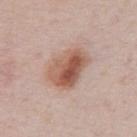{
  "biopsy_status": "not biopsied; imaged during a skin examination",
  "image": {
    "source": "total-body photography crop",
    "field_of_view_mm": 15
  },
  "site": "chest",
  "automated_metrics": {
    "area_mm2_approx": 14.0,
    "eccentricity": 0.7,
    "shape_asymmetry": 0.2,
    "nevus_likeness_0_100": 95,
    "lesion_detection_confidence_0_100": 100
  },
  "lighting": "white-light",
  "patient": {
    "sex": "male",
    "age_approx": 75
  },
  "lesion_size": {
    "long_diameter_mm_approx": 5.0
  }
}